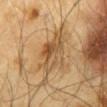No biopsy was performed on this lesion — it was imaged during a full skin examination and was not determined to be concerning.
The patient is a male aged 63–67.
A 15 mm crop from a total-body photograph taken for skin-cancer surveillance.
The lesion is located on the mid back.